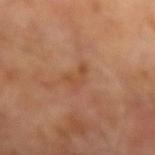biopsy_status: not biopsied; imaged during a skin examination
site: left forearm
image:
  source: total-body photography crop
  field_of_view_mm: 15
patient:
  sex: male
  age_approx: 70